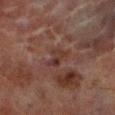This lesion was catalogued during total-body skin photography and was not selected for biopsy. On the left lower leg. A roughly 15 mm field-of-view crop from a total-body skin photograph. Measured at roughly 3 mm in maximum diameter. An algorithmic analysis of the crop reported a lesion-to-skin contrast of about 6 (normalized; higher = more distinct). The analysis additionally found a border-irregularity rating of about 5.5/10, a color-variation rating of about 5/10, and radial color variation of about 2. The software also gave a nevus-likeness score of about 0/100. Imaged with cross-polarized lighting. The subject is a male aged approximately 70.